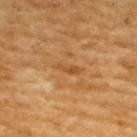The lesion was tiled from a total-body skin photograph and was not biopsied.
A lesion tile, about 15 mm wide, cut from a 3D total-body photograph.
Imaged with cross-polarized lighting.
A male subject aged approximately 60.
Located on the upper back.